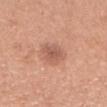patient = female, aged 33 to 37
TBP lesion metrics = a border-irregularity rating of about 2/10, a color-variation rating of about 2.5/10, and radial color variation of about 0.5
image source = 15 mm crop, total-body photography
lesion diameter = about 3.5 mm
site = the head or neck